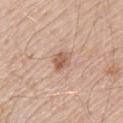Notes:
- notes · catalogued during a skin exam; not biopsied
- patient · male, aged 68 to 72
- anatomic site · the upper back
- image · ~15 mm tile from a whole-body skin photo